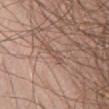biopsy status: imaged on a skin check; not biopsied
image-analysis metrics: an outline eccentricity of about 0.95 (0 = round, 1 = elongated) and two-axis asymmetry of about 0.35; a lesion color around L≈52 a*≈18 b*≈25 in CIELAB, a lesion–skin lightness drop of about 7, and a lesion-to-skin contrast of about 5 (normalized; higher = more distinct)
image: 15 mm crop, total-body photography
patient: male, approximately 65 years of age
size: about 3 mm
tile lighting: white-light
anatomic site: the abdomen